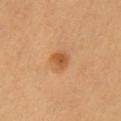Part of a total-body skin-imaging series; this lesion was reviewed on a skin check and was not flagged for biopsy. From the front of the torso. A male patient aged approximately 60. A region of skin cropped from a whole-body photographic capture, roughly 15 mm wide.